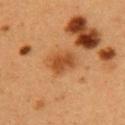The lesion was photographed on a routine skin check and not biopsied; there is no pathology result. A close-up tile cropped from a whole-body skin photograph, about 15 mm across. The lesion is on the arm. A female subject, aged approximately 40.